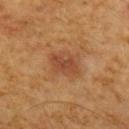Case summary:
* biopsy status: no biopsy performed (imaged during a skin exam)
* body site: the mid back
* size: ≈3.5 mm
* image-analysis metrics: a border-irregularity index near 3.5/10 and a within-lesion color-variation index near 2/10
* patient: male, aged approximately 60
* tile lighting: cross-polarized
* acquisition: ~15 mm tile from a whole-body skin photo